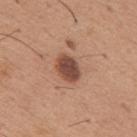The lesion was photographed on a routine skin check and not biopsied; there is no pathology result. On the upper back. About 3.5 mm across. A region of skin cropped from a whole-body photographic capture, roughly 15 mm wide. Captured under white-light illumination. The patient is a male aged approximately 65.